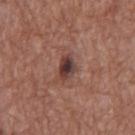  biopsy_status: not biopsied; imaged during a skin examination
  patient:
    sex: male
    age_approx: 75
  image:
    source: total-body photography crop
    field_of_view_mm: 15
  automated_metrics:
    color_variation_0_10: 5.0
    nevus_likeness_0_100: 15
    lesion_detection_confidence_0_100: 100
  lesion_size:
    long_diameter_mm_approx: 3.0
  site: chest
  lighting: white-light